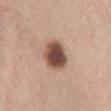notes: no biopsy performed (imaged during a skin exam) | TBP lesion metrics: a lesion area of about 11 mm²; a border-irregularity rating of about 1/10 and radial color variation of about 1.5 | patient: female, aged around 35 | image source: total-body-photography crop, ~15 mm field of view | location: the front of the torso.